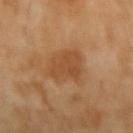This lesion was catalogued during total-body skin photography and was not selected for biopsy.
Cropped from a whole-body photographic skin survey; the tile spans about 15 mm.
The lesion's longest dimension is about 5.5 mm.
The lesion is on the arm.
The total-body-photography lesion software estimated an area of roughly 12 mm² and an eccentricity of roughly 0.8.
The tile uses cross-polarized illumination.
A female patient, in their 70s.